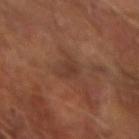Clinical impression:
The lesion was tiled from a total-body skin photograph and was not biopsied.
Background:
From the arm. This image is a 15 mm lesion crop taken from a total-body photograph. A male patient aged 63–67. About 2.5 mm across. Imaged with cross-polarized lighting.